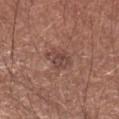Clinical impression:
The lesion was tiled from a total-body skin photograph and was not biopsied.
Acquisition and patient details:
Cropped from a total-body skin-imaging series; the visible field is about 15 mm. The tile uses white-light illumination. A male subject, in their mid-60s. Located on the left forearm.